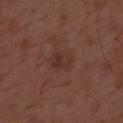Q: Was a biopsy performed?
A: no biopsy performed (imaged during a skin exam)
Q: Lesion size?
A: about 2.5 mm
Q: What are the patient's age and sex?
A: male, in their 50s
Q: How was the tile lit?
A: white-light illumination
Q: Automated lesion metrics?
A: a lesion area of about 5 mm² and a shape eccentricity near 0.6; a border-irregularity index near 2.5/10 and radial color variation of about 1
Q: What is the imaging modality?
A: total-body-photography crop, ~15 mm field of view